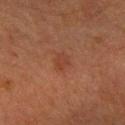  biopsy_status: not biopsied; imaged during a skin examination
  image:
    source: total-body photography crop
    field_of_view_mm: 15
  site: right forearm
  patient:
    sex: male
    age_approx: 50